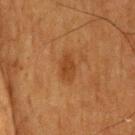This lesion was catalogued during total-body skin photography and was not selected for biopsy. The recorded lesion diameter is about 3.5 mm. A 15 mm crop from a total-body photograph taken for skin-cancer surveillance. The tile uses cross-polarized illumination. The patient is a male approximately 75 years of age. From the head or neck. The total-body-photography lesion software estimated an area of roughly 5 mm² and a shape eccentricity near 0.8.